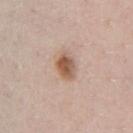The lesion was tiled from a total-body skin photograph and was not biopsied. Cropped from a total-body skin-imaging series; the visible field is about 15 mm. From the chest. An algorithmic analysis of the crop reported a shape-asymmetry score of about 0.2 (0 = symmetric). Measured at roughly 3.5 mm in maximum diameter. A male subject roughly 40 years of age.